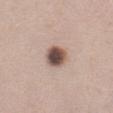{
  "biopsy_status": "not biopsied; imaged during a skin examination",
  "lighting": "white-light",
  "image": {
    "source": "total-body photography crop",
    "field_of_view_mm": 15
  },
  "patient": {
    "sex": "female",
    "age_approx": 40
  },
  "site": "abdomen",
  "automated_metrics": {
    "cielab_L": 51,
    "cielab_a": 16,
    "cielab_b": 23,
    "vs_skin_darker_L": 18.0,
    "vs_skin_contrast_norm": 12.0,
    "color_variation_0_10": 7.0,
    "peripheral_color_asymmetry": 1.5
  }
}